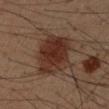Impression:
No biopsy was performed on this lesion — it was imaged during a full skin examination and was not determined to be concerning.
Clinical summary:
Cropped from a whole-body photographic skin survey; the tile spans about 15 mm. From the right upper arm. A male subject about 50 years old. The tile uses cross-polarized illumination. Automated tile analysis of the lesion measured a detector confidence of about 100 out of 100 that the crop contains a lesion.